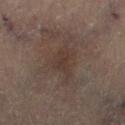<tbp_lesion>
<biopsy_status>not biopsied; imaged during a skin examination</biopsy_status>
<image>
  <source>total-body photography crop</source>
  <field_of_view_mm>15</field_of_view_mm>
</image>
<lesion_size>
  <long_diameter_mm_approx>3.5</long_diameter_mm_approx>
</lesion_size>
<lighting>cross-polarized</lighting>
<site>left leg</site>
<patient>
  <sex>female</sex>
  <age_approx>80</age_approx>
</patient>
</tbp_lesion>